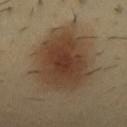notes: total-body-photography surveillance lesion; no biopsy | subject: female, aged approximately 40 | automated metrics: a lesion area of about 47 mm², a shape eccentricity near 0.6, and a symmetry-axis asymmetry near 0.15; a mean CIELAB color near L≈41 a*≈14 b*≈29 and a normalized lesion–skin contrast near 9 | location: the chest | lesion diameter: about 9 mm | acquisition: ~15 mm tile from a whole-body skin photo | tile lighting: cross-polarized.